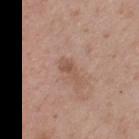{
  "biopsy_status": "not biopsied; imaged during a skin examination",
  "lighting": "white-light",
  "automated_metrics": {
    "vs_skin_contrast_norm": 5.5,
    "border_irregularity_0_10": 4.0,
    "color_variation_0_10": 0.5,
    "peripheral_color_asymmetry": 0.0,
    "nevus_likeness_0_100": 0,
    "lesion_detection_confidence_0_100": 100
  },
  "site": "upper back",
  "image": {
    "source": "total-body photography crop",
    "field_of_view_mm": 15
  },
  "patient": {
    "sex": "male",
    "age_approx": 40
  },
  "lesion_size": {
    "long_diameter_mm_approx": 3.0
  }
}